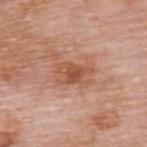<tbp_lesion>
<biopsy_status>not biopsied; imaged during a skin examination</biopsy_status>
<lesion_size>
  <long_diameter_mm_approx>4.0</long_diameter_mm_approx>
</lesion_size>
<lighting>white-light</lighting>
<automated_metrics>
  <border_irregularity_0_10>3.0</border_irregularity_0_10>
  <peripheral_color_asymmetry>1.0</peripheral_color_asymmetry>
</automated_metrics>
<image>
  <source>total-body photography crop</source>
  <field_of_view_mm>15</field_of_view_mm>
</image>
<patient>
  <sex>male</sex>
  <age_approx>60</age_approx>
</patient>
<site>upper back</site>
</tbp_lesion>